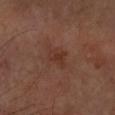follow-up — imaged on a skin check; not biopsied | image source — 15 mm crop, total-body photography | anatomic site — the right forearm | diameter — ≈3 mm | patient — male, aged 68–72.